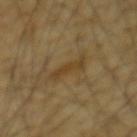Captured during whole-body skin photography for melanoma surveillance; the lesion was not biopsied.
A 15 mm close-up extracted from a 3D total-body photography capture.
The total-body-photography lesion software estimated an area of roughly 5 mm², an outline eccentricity of about 0.95 (0 = round, 1 = elongated), and two-axis asymmetry of about 0.45. The software also gave a classifier nevus-likeness of about 0/100 and lesion-presence confidence of about 75/100.
A male subject in their mid- to late 60s.
Longest diameter approximately 4 mm.
Imaged with cross-polarized lighting.
From the mid back.